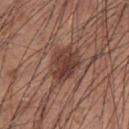The lesion was tiled from a total-body skin photograph and was not biopsied. A lesion tile, about 15 mm wide, cut from a 3D total-body photograph. The lesion is on the front of the torso. Imaged with white-light lighting. The subject is a male aged around 60.